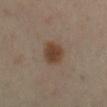A roughly 15 mm field-of-view crop from a total-body skin photograph.
The patient is a female about 40 years old.
Imaged with cross-polarized lighting.
Located on the left leg.